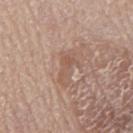Findings:
* lesion size — ~3.5 mm (longest diameter)
* patient — male, aged 78–82
* illumination — white-light
* image source — ~15 mm crop, total-body skin-cancer survey
* image-analysis metrics — a footprint of about 3.5 mm² and two-axis asymmetry of about 0.55; an average lesion color of about L≈54 a*≈19 b*≈28 (CIELAB), a lesion–skin lightness drop of about 7, and a normalized border contrast of about 5.5; a color-variation rating of about 0.5/10 and peripheral color asymmetry of about 0; a nevus-likeness score of about 0/100 and a lesion-detection confidence of about 95/100
* anatomic site — the back